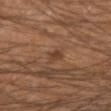This lesion was catalogued during total-body skin photography and was not selected for biopsy.
A male patient aged approximately 45.
This is a cross-polarized tile.
An algorithmic analysis of the crop reported a lesion color around L≈39 a*≈19 b*≈29 in CIELAB, roughly 7 lightness units darker than nearby skin, and a normalized lesion–skin contrast near 6.5. It also reported a classifier nevus-likeness of about 25/100 and a detector confidence of about 100 out of 100 that the crop contains a lesion.
A roughly 15 mm field-of-view crop from a total-body skin photograph.
About 2.5 mm across.
The lesion is on the arm.